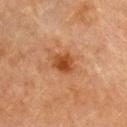notes — no biopsy performed (imaged during a skin exam) | subject — female, aged 58–62 | image-analysis metrics — an area of roughly 5 mm² and a shape eccentricity near 0.5; border irregularity of about 2 on a 0–10 scale, a within-lesion color-variation index near 3/10, and radial color variation of about 1; a nevus-likeness score of about 90/100 and a detector confidence of about 100 out of 100 that the crop contains a lesion | site — the chest | image source — total-body-photography crop, ~15 mm field of view | tile lighting — cross-polarized illumination.